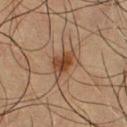Clinical impression:
The lesion was tiled from a total-body skin photograph and was not biopsied.
Context:
Cropped from a total-body skin-imaging series; the visible field is about 15 mm. The lesion is located on the chest. A male subject, aged 58 to 62.